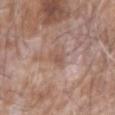• lighting · white-light illumination
• patient · male, aged 73–77
• TBP lesion metrics · a nevus-likeness score of about 0/100 and lesion-presence confidence of about 85/100
• site · the right forearm
• image source · ~15 mm crop, total-body skin-cancer survey